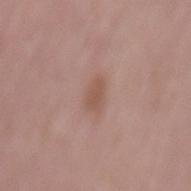Impression: Recorded during total-body skin imaging; not selected for excision or biopsy. Clinical summary: A roughly 15 mm field-of-view crop from a total-body skin photograph. Captured under white-light illumination. On the mid back. The subject is a female in their mid-60s.